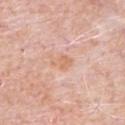notes = catalogued during a skin exam; not biopsied
subject = male, in their 80s
anatomic site = the chest
size = ~2.5 mm (longest diameter)
acquisition = ~15 mm crop, total-body skin-cancer survey